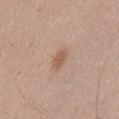Impression: Recorded during total-body skin imaging; not selected for excision or biopsy. Context: The subject is a male approximately 50 years of age. Captured under white-light illumination. The lesion is on the front of the torso. The recorded lesion diameter is about 2.5 mm. A lesion tile, about 15 mm wide, cut from a 3D total-body photograph.